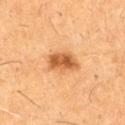biopsy_status: not biopsied; imaged during a skin examination
automated_metrics:
  area_mm2_approx: 8.5
  eccentricity: 0.8
  shape_asymmetry: 0.2
  cielab_L: 57
  cielab_a: 25
  cielab_b: 42
  vs_skin_darker_L: 14.0
  vs_skin_contrast_norm: 9.0
  border_irregularity_0_10: 2.0
  color_variation_0_10: 4.5
  peripheral_color_asymmetry: 1.5
lesion_size:
  long_diameter_mm_approx: 4.0
image:
  source: total-body photography crop
  field_of_view_mm: 15
lighting: cross-polarized
site: leg
patient:
  sex: male
  age_approx: 60